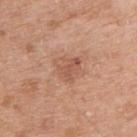biopsy status: total-body-photography surveillance lesion; no biopsy | anatomic site: the right upper arm | lighting: white-light | patient: male, approximately 65 years of age | diameter: ~3.5 mm (longest diameter) | imaging modality: 15 mm crop, total-body photography.